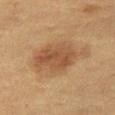Impression:
Captured during whole-body skin photography for melanoma surveillance; the lesion was not biopsied.
Background:
A 15 mm crop from a total-body photograph taken for skin-cancer surveillance. The tile uses cross-polarized illumination. The lesion is on the right thigh. The patient is a female roughly 55 years of age. The total-body-photography lesion software estimated an outline eccentricity of about 0.75 (0 = round, 1 = elongated). The analysis additionally found a lesion color around L≈42 a*≈16 b*≈29 in CIELAB, roughly 8 lightness units darker than nearby skin, and a normalized border contrast of about 7. The analysis additionally found a classifier nevus-likeness of about 60/100 and a detector confidence of about 100 out of 100 that the crop contains a lesion. About 7 mm across.